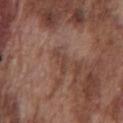Captured during whole-body skin photography for melanoma surveillance; the lesion was not biopsied. Automated tile analysis of the lesion measured a footprint of about 3 mm², an eccentricity of roughly 0.9, and a shape-asymmetry score of about 0.4 (0 = symmetric). The analysis additionally found border irregularity of about 4.5 on a 0–10 scale, a within-lesion color-variation index near 0/10, and radial color variation of about 0. It also reported a nevus-likeness score of about 0/100 and a detector confidence of about 90 out of 100 that the crop contains a lesion. A male subject aged 73–77. A 15 mm close-up extracted from a 3D total-body photography capture. This is a white-light tile. The lesion's longest dimension is about 3 mm. On the front of the torso.